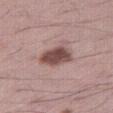biopsy_status: not biopsied; imaged during a skin examination
site: right thigh
patient:
  sex: male
  age_approx: 50
automated_metrics:
  area_mm2_approx: 9.0
  eccentricity: 0.65
  shape_asymmetry: 0.2
  cielab_L: 47
  cielab_a: 20
  cielab_b: 20
  vs_skin_darker_L: 16.0
  vs_skin_contrast_norm: 11.0
image:
  source: total-body photography crop
  field_of_view_mm: 15
lighting: white-light